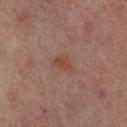Impression: The lesion was photographed on a routine skin check and not biopsied; there is no pathology result. Clinical summary: Located on the left thigh. Captured under cross-polarized illumination. Measured at roughly 2.5 mm in maximum diameter. Automated tile analysis of the lesion measured an area of roughly 3.5 mm², an outline eccentricity of about 0.75 (0 = round, 1 = elongated), and a symmetry-axis asymmetry near 0.35. It also reported a classifier nevus-likeness of about 5/100. The patient is a female aged 48–52. This image is a 15 mm lesion crop taken from a total-body photograph.